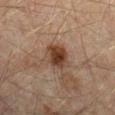  biopsy_status: not biopsied; imaged during a skin examination
  patient:
    sex: male
    age_approx: 45
  image:
    source: total-body photography crop
    field_of_view_mm: 15
  site: left forearm